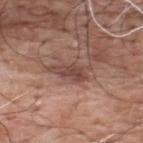Q: Was this lesion biopsied?
A: total-body-photography surveillance lesion; no biopsy
Q: Patient demographics?
A: male, aged around 60
Q: How was the tile lit?
A: white-light
Q: Lesion location?
A: the upper back
Q: What is the lesion's diameter?
A: ≈4.5 mm
Q: What did automated image analysis measure?
A: a border-irregularity rating of about 4.5/10, a color-variation rating of about 3.5/10, and a peripheral color-asymmetry measure near 1
Q: What is the imaging modality?
A: ~15 mm tile from a whole-body skin photo